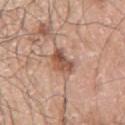Impression: This lesion was catalogued during total-body skin photography and was not selected for biopsy. Background: On the arm. Measured at roughly 4 mm in maximum diameter. The tile uses white-light illumination. A male patient, approximately 70 years of age. A close-up tile cropped from a whole-body skin photograph, about 15 mm across.